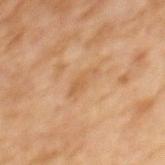workup: catalogued during a skin exam; not biopsied
acquisition: ~15 mm tile from a whole-body skin photo
anatomic site: the upper back
subject: female, in their 60s
illumination: cross-polarized